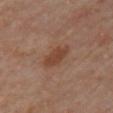Part of a total-body skin-imaging series; this lesion was reviewed on a skin check and was not flagged for biopsy. A region of skin cropped from a whole-body photographic capture, roughly 15 mm wide. The patient is a female approximately 50 years of age. The lesion is located on the right upper arm. Longest diameter approximately 4 mm. This is a cross-polarized tile. Automated tile analysis of the lesion measured a symmetry-axis asymmetry near 0.2.